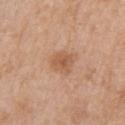Impression: Part of a total-body skin-imaging series; this lesion was reviewed on a skin check and was not flagged for biopsy. Background: Cropped from a total-body skin-imaging series; the visible field is about 15 mm. The tile uses white-light illumination. The subject is a female in their mid- to late 50s. Located on the right upper arm. Longest diameter approximately 3 mm.